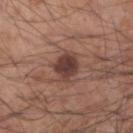biopsy status = no biopsy performed (imaged during a skin exam) | lesion size = ≈4 mm | image = ~15 mm crop, total-body skin-cancer survey | patient = male, aged 53 to 57 | body site = the arm | illumination = white-light illumination.